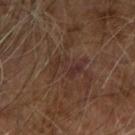This lesion was catalogued during total-body skin photography and was not selected for biopsy.